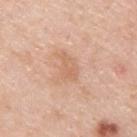Imaged during a routine full-body skin examination; the lesion was not biopsied and no histopathology is available.
On the upper back.
The subject is a male in their mid- to late 40s.
A lesion tile, about 15 mm wide, cut from a 3D total-body photograph.